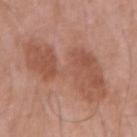The lesion was photographed on a routine skin check and not biopsied; there is no pathology result.
A roughly 15 mm field-of-view crop from a total-body skin photograph.
Captured under white-light illumination.
The patient is a male aged 63 to 67.
About 10.5 mm across.
The lesion is on the right upper arm.
Automated tile analysis of the lesion measured a border-irregularity rating of about 7.5/10 and internal color variation of about 3.5 on a 0–10 scale.